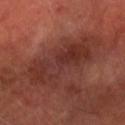Assessment:
The lesion was tiled from a total-body skin photograph and was not biopsied.
Context:
A male patient aged around 65. The lesion-visualizer software estimated a lesion color around L≈30 a*≈23 b*≈23 in CIELAB, roughly 7 lightness units darker than nearby skin, and a normalized lesion–skin contrast near 7. It also reported a lesion-detection confidence of about 85/100. Located on the left forearm. This image is a 15 mm lesion crop taken from a total-body photograph.